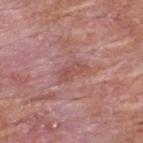notes: imaged on a skin check; not biopsied | imaging modality: 15 mm crop, total-body photography | diameter: ≈3.5 mm | image-analysis metrics: a lesion color around L≈51 a*≈25 b*≈24 in CIELAB and roughly 7 lightness units darker than nearby skin; a border-irregularity rating of about 4/10, internal color variation of about 2 on a 0–10 scale, and radial color variation of about 0.5; a classifier nevus-likeness of about 0/100 and a detector confidence of about 100 out of 100 that the crop contains a lesion | lighting: white-light | subject: male, about 55 years old | body site: the upper back.